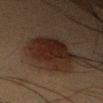follow-up: total-body-photography surveillance lesion; no biopsy | image-analysis metrics: a footprint of about 21 mm², a shape eccentricity near 0.75, and two-axis asymmetry of about 0.2; a border-irregularity index near 3/10, a within-lesion color-variation index near 3.5/10, and peripheral color asymmetry of about 1; a classifier nevus-likeness of about 100/100 | body site: the arm | acquisition: ~15 mm crop, total-body skin-cancer survey | tile lighting: cross-polarized | subject: male, in their 50s.